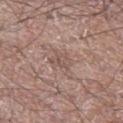Part of a total-body skin-imaging series; this lesion was reviewed on a skin check and was not flagged for biopsy. A 15 mm crop from a total-body photograph taken for skin-cancer surveillance. Measured at roughly 2.5 mm in maximum diameter. A male subject, aged 63–67. Automated image analysis of the tile measured a footprint of about 3.5 mm², an eccentricity of roughly 0.75, and a symmetry-axis asymmetry near 0.4. It also reported a mean CIELAB color near L≈52 a*≈18 b*≈23 and about 7 CIELAB-L* units darker than the surrounding skin. And it measured a color-variation rating of about 2.5/10 and peripheral color asymmetry of about 1. From the leg. The tile uses white-light illumination.